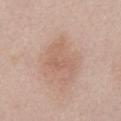notes = imaged on a skin check; not biopsied
patient = male, in their mid-50s
size = ≈5.5 mm
illumination = white-light illumination
anatomic site = the abdomen
image = ~15 mm tile from a whole-body skin photo
image-analysis metrics = a footprint of about 12 mm² and a symmetry-axis asymmetry near 0.4; an automated nevus-likeness rating near 5 out of 100 and a detector confidence of about 100 out of 100 that the crop contains a lesion